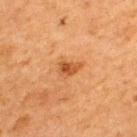Findings:
– biopsy status · imaged on a skin check; not biopsied
– location · the upper back
– subject · male, approximately 65 years of age
– lesion diameter · about 3 mm
– image source · ~15 mm tile from a whole-body skin photo